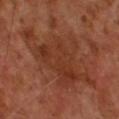This is a cross-polarized tile. A 15 mm crop from a total-body photograph taken for skin-cancer surveillance. Approximately 11 mm at its widest. A male patient aged approximately 65. Automated image analysis of the tile measured a mean CIELAB color near L≈35 a*≈24 b*≈30, roughly 7 lightness units darker than nearby skin, and a lesion-to-skin contrast of about 6.5 (normalized; higher = more distinct). The software also gave a border-irregularity index near 7.5/10 and a peripheral color-asymmetry measure near 1. The software also gave a lesion-detection confidence of about 95/100.